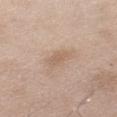Q: Was this lesion biopsied?
A: no biopsy performed (imaged during a skin exam)
Q: What is the anatomic site?
A: the left upper arm
Q: Automated lesion metrics?
A: a color-variation rating of about 1/10 and radial color variation of about 0.5
Q: What is the lesion's diameter?
A: ≈2.5 mm
Q: Patient demographics?
A: male, about 50 years old
Q: How was this image acquired?
A: ~15 mm tile from a whole-body skin photo
Q: What lighting was used for the tile?
A: white-light